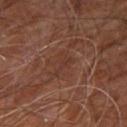  biopsy_status: not biopsied; imaged during a skin examination
  image:
    source: total-body photography crop
    field_of_view_mm: 15
  automated_metrics:
    border_irregularity_0_10: 7.0
    color_variation_0_10: 0.0
    peripheral_color_asymmetry: 0.0
    lesion_detection_confidence_0_100: 90
  patient:
    sex: male
    age_approx: 60
  site: right leg
  lesion_size:
    long_diameter_mm_approx: 3.0